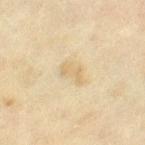Case summary:
• workup: catalogued during a skin exam; not biopsied
• tile lighting: cross-polarized
• image: ~15 mm tile from a whole-body skin photo
• lesion diameter: ≈3 mm
• patient: female, roughly 55 years of age
• anatomic site: the right thigh